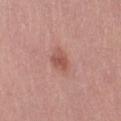A female patient aged around 65.
A 15 mm close-up extracted from a 3D total-body photography capture.
The lesion is located on the left thigh.